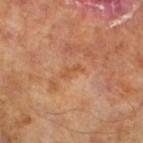Background: The tile uses cross-polarized illumination. A 15 mm close-up extracted from a 3D total-body photography capture. Automated image analysis of the tile measured an average lesion color of about L≈52 a*≈23 b*≈37 (CIELAB), a lesion–skin lightness drop of about 6, and a lesion-to-skin contrast of about 5.5 (normalized; higher = more distinct). And it measured an automated nevus-likeness rating near 0 out of 100 and lesion-presence confidence of about 100/100. The patient is a male aged 63–67. Longest diameter approximately 2.5 mm.